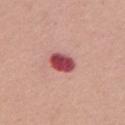Acquisition and patient details:
The recorded lesion diameter is about 3 mm. The lesion is located on the chest. An algorithmic analysis of the crop reported a mean CIELAB color near L≈49 a*≈35 b*≈22, roughly 19 lightness units darker than nearby skin, and a normalized lesion–skin contrast near 12.5. The software also gave border irregularity of about 1 on a 0–10 scale, a within-lesion color-variation index near 5/10, and peripheral color asymmetry of about 1.5. The analysis additionally found a lesion-detection confidence of about 100/100. A 15 mm close-up extracted from a 3D total-body photography capture. A female subject approximately 55 years of age. Captured under white-light illumination.